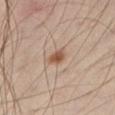{
  "biopsy_status": "not biopsied; imaged during a skin examination",
  "site": "right thigh",
  "patient": {
    "sex": "male",
    "age_approx": 45
  },
  "image": {
    "source": "total-body photography crop",
    "field_of_view_mm": 15
  },
  "lesion_size": {
    "long_diameter_mm_approx": 2.5
  },
  "lighting": "cross-polarized"
}